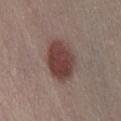follow-up=imaged on a skin check; not biopsied
subject=female, aged 28–32
imaging modality=~15 mm tile from a whole-body skin photo
site=the abdomen